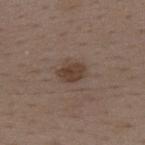| feature | finding |
|---|---|
| follow-up | total-body-photography surveillance lesion; no biopsy |
| patient | female, roughly 35 years of age |
| lighting | white-light |
| image | ~15 mm tile from a whole-body skin photo |
| lesion diameter | ≈3.5 mm |
| automated metrics | a footprint of about 6 mm², an outline eccentricity of about 0.7 (0 = round, 1 = elongated), and a shape-asymmetry score of about 0.3 (0 = symmetric); a border-irregularity rating of about 2.5/10 |
| location | the mid back |